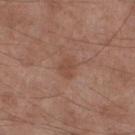Assessment: No biopsy was performed on this lesion — it was imaged during a full skin examination and was not determined to be concerning. Clinical summary: The recorded lesion diameter is about 2.5 mm. The lesion is located on the right lower leg. A 15 mm crop from a total-body photograph taken for skin-cancer surveillance. An algorithmic analysis of the crop reported a lesion area of about 4 mm², an eccentricity of roughly 0.65, and a shape-asymmetry score of about 0.3 (0 = symmetric). And it measured border irregularity of about 3 on a 0–10 scale and radial color variation of about 0.5. A male patient, roughly 55 years of age. Captured under white-light illumination.